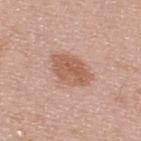<case>
  <biopsy_status>not biopsied; imaged during a skin examination</biopsy_status>
  <site>back</site>
  <image>
    <source>total-body photography crop</source>
    <field_of_view_mm>15</field_of_view_mm>
  </image>
  <lighting>white-light</lighting>
  <patient>
    <sex>male</sex>
    <age_approx>45</age_approx>
  </patient>
</case>